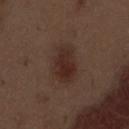{
  "biopsy_status": "not biopsied; imaged during a skin examination",
  "patient": {
    "sex": "male",
    "age_approx": 70
  },
  "lighting": "white-light",
  "image": {
    "source": "total-body photography crop",
    "field_of_view_mm": 15
  },
  "automated_metrics": {
    "border_irregularity_0_10": 2.0,
    "peripheral_color_asymmetry": 1.0,
    "nevus_likeness_0_100": 90,
    "lesion_detection_confidence_0_100": 100
  },
  "site": "abdomen",
  "lesion_size": {
    "long_diameter_mm_approx": 5.0
  }
}